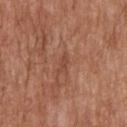The lesion was tiled from a total-body skin photograph and was not biopsied. A region of skin cropped from a whole-body photographic capture, roughly 15 mm wide. An algorithmic analysis of the crop reported a lesion color around L≈46 a*≈25 b*≈30 in CIELAB and a normalized lesion–skin contrast near 5.5. The analysis additionally found a border-irregularity index near 3.5/10 and radial color variation of about 0. It also reported an automated nevus-likeness rating near 0 out of 100 and a detector confidence of about 85 out of 100 that the crop contains a lesion. This is a white-light tile. The lesion is on the upper back. A male patient aged around 60.